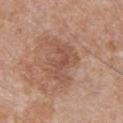No biopsy was performed on this lesion — it was imaged during a full skin examination and was not determined to be concerning. From the front of the torso. A close-up tile cropped from a whole-body skin photograph, about 15 mm across. Captured under white-light illumination. The recorded lesion diameter is about 5.5 mm. An algorithmic analysis of the crop reported border irregularity of about 5.5 on a 0–10 scale and peripheral color asymmetry of about 1. The software also gave a classifier nevus-likeness of about 0/100 and a detector confidence of about 100 out of 100 that the crop contains a lesion. The patient is a male aged around 30.